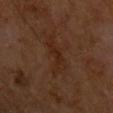biopsy status: no biopsy performed (imaged during a skin exam); size: ~5 mm (longest diameter); acquisition: ~15 mm crop, total-body skin-cancer survey; tile lighting: cross-polarized illumination; subject: male, aged 63–67; body site: the left upper arm.